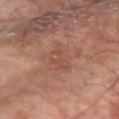Clinical impression:
Imaged during a routine full-body skin examination; the lesion was not biopsied and no histopathology is available.
Background:
This image is a 15 mm lesion crop taken from a total-body photograph. Automated image analysis of the tile measured a lesion area of about 6 mm², a shape eccentricity near 0.9, and two-axis asymmetry of about 0.45. And it measured peripheral color asymmetry of about 0.5. The software also gave a nevus-likeness score of about 0/100. From the left forearm. Approximately 4.5 mm at its widest. The patient is a male aged around 65.